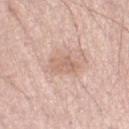No biopsy was performed on this lesion — it was imaged during a full skin examination and was not determined to be concerning.
Imaged with white-light lighting.
The lesion is on the left thigh.
A 15 mm crop from a total-body photograph taken for skin-cancer surveillance.
A female patient aged 58 to 62.
The lesion-visualizer software estimated border irregularity of about 3.5 on a 0–10 scale, a within-lesion color-variation index near 2/10, and a peripheral color-asymmetry measure near 0.5. The software also gave a classifier nevus-likeness of about 0/100.
About 3.5 mm across.